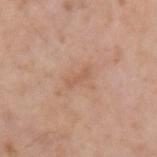Recorded during total-body skin imaging; not selected for excision or biopsy.
On the left upper arm.
The total-body-photography lesion software estimated a border-irregularity rating of about 4.5/10 and radial color variation of about 0.
Imaged with white-light lighting.
The subject is a male aged around 60.
A close-up tile cropped from a whole-body skin photograph, about 15 mm across.
Approximately 2.5 mm at its widest.